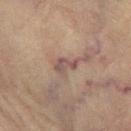Q: Lesion location?
A: the left thigh
Q: Patient demographics?
A: female, about 80 years old
Q: What kind of image is this?
A: ~15 mm crop, total-body skin-cancer survey
Q: Automated lesion metrics?
A: a lesion color around L≈43 a*≈15 b*≈19 in CIELAB, roughly 7 lightness units darker than nearby skin, and a normalized lesion–skin contrast near 6.5